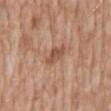imaging modality: ~15 mm tile from a whole-body skin photo | automated metrics: lesion-presence confidence of about 100/100 | site: the abdomen | diameter: ~3 mm (longest diameter) | subject: male, aged 58–62.